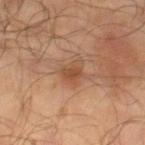workup: catalogued during a skin exam; not biopsied | size: ~3 mm (longest diameter) | location: the leg | image source: total-body-photography crop, ~15 mm field of view | patient: male, aged around 65 | automated metrics: a nevus-likeness score of about 80/100 and lesion-presence confidence of about 100/100 | lighting: cross-polarized illumination.